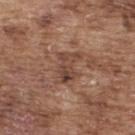notes: total-body-photography surveillance lesion; no biopsy
subject: male, aged 73 to 77
image source: 15 mm crop, total-body photography
site: the upper back
lesion diameter: ~4.5 mm (longest diameter)
tile lighting: white-light illumination